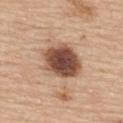| key | value |
|---|---|
| notes | imaged on a skin check; not biopsied |
| automated lesion analysis | a lesion area of about 17 mm², an eccentricity of roughly 0.6, and a symmetry-axis asymmetry near 0.15; a lesion color around L≈50 a*≈21 b*≈28 in CIELAB, roughly 19 lightness units darker than nearby skin, and a normalized lesion–skin contrast near 12.5; a classifier nevus-likeness of about 65/100 and lesion-presence confidence of about 100/100 |
| lesion diameter | about 5.5 mm |
| image | total-body-photography crop, ~15 mm field of view |
| body site | the upper back |
| subject | female, about 65 years old |